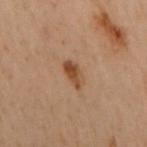This lesion was catalogued during total-body skin photography and was not selected for biopsy. The lesion is on the upper back. Cropped from a total-body skin-imaging series; the visible field is about 15 mm. Imaged with cross-polarized lighting. The subject is a female aged 58 to 62. About 3 mm across. The lesion-visualizer software estimated an area of roughly 4.5 mm², an outline eccentricity of about 0.8 (0 = round, 1 = elongated), and a symmetry-axis asymmetry near 0.2.